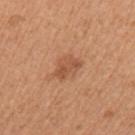Q: Was a biopsy performed?
A: no biopsy performed (imaged during a skin exam)
Q: How was the tile lit?
A: white-light
Q: What is the lesion's diameter?
A: ~3.5 mm (longest diameter)
Q: What are the patient's age and sex?
A: female, roughly 50 years of age
Q: How was this image acquired?
A: total-body-photography crop, ~15 mm field of view
Q: Where on the body is the lesion?
A: the left upper arm
Q: Automated lesion metrics?
A: a lesion area of about 5.5 mm², an eccentricity of roughly 0.75, and two-axis asymmetry of about 0.4; an average lesion color of about L≈53 a*≈25 b*≈35 (CIELAB) and about 9 CIELAB-L* units darker than the surrounding skin; an automated nevus-likeness rating near 40 out of 100